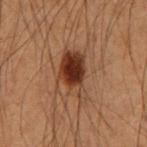<tbp_lesion>
<biopsy_status>not biopsied; imaged during a skin examination</biopsy_status>
<automated_metrics>
  <cielab_L>30</cielab_L>
  <cielab_a>18</cielab_a>
  <cielab_b>26</cielab_b>
  <vs_skin_darker_L>11.0</vs_skin_darker_L>
  <vs_skin_contrast_norm>10.5</vs_skin_contrast_norm>
  <nevus_likeness_0_100>100</nevus_likeness_0_100>
  <lesion_detection_confidence_0_100>100</lesion_detection_confidence_0_100>
</automated_metrics>
<patient>
  <sex>male</sex>
  <age_approx>55</age_approx>
</patient>
<site>arm</site>
<image>
  <source>total-body photography crop</source>
  <field_of_view_mm>15</field_of_view_mm>
</image>
<lesion_size>
  <long_diameter_mm_approx>5.5</long_diameter_mm_approx>
</lesion_size>
</tbp_lesion>